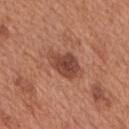Q: What is the lesion's diameter?
A: ~4.5 mm (longest diameter)
Q: What lighting was used for the tile?
A: white-light
Q: What did automated image analysis measure?
A: a lesion area of about 9 mm², an outline eccentricity of about 0.8 (0 = round, 1 = elongated), and a shape-asymmetry score of about 0.25 (0 = symmetric); a lesion color around L≈45 a*≈25 b*≈29 in CIELAB, roughly 12 lightness units darker than nearby skin, and a lesion-to-skin contrast of about 9 (normalized; higher = more distinct); an automated nevus-likeness rating near 55 out of 100
Q: Lesion location?
A: the back
Q: What kind of image is this?
A: ~15 mm crop, total-body skin-cancer survey
Q: What are the patient's age and sex?
A: male, aged approximately 65